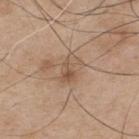Impression:
Recorded during total-body skin imaging; not selected for excision or biopsy.
Acquisition and patient details:
About 3.5 mm across. The subject is a male in their mid-70s. An algorithmic analysis of the crop reported radial color variation of about 2.5. A 15 mm close-up tile from a total-body photography series done for melanoma screening. On the upper back. The tile uses white-light illumination.